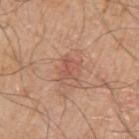Q: Was this lesion biopsied?
A: total-body-photography surveillance lesion; no biopsy
Q: Lesion location?
A: the right upper arm
Q: What are the patient's age and sex?
A: male, aged 78 to 82
Q: How was this image acquired?
A: 15 mm crop, total-body photography